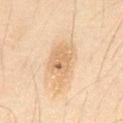The lesion was tiled from a total-body skin photograph and was not biopsied. Located on the abdomen. About 5.5 mm across. A close-up tile cropped from a whole-body skin photograph, about 15 mm across. Captured under cross-polarized illumination. A male subject, about 65 years old.